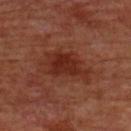workup = total-body-photography surveillance lesion; no biopsy | lesion size = ~6 mm (longest diameter) | image = total-body-photography crop, ~15 mm field of view | patient = male, aged 58 to 62 | anatomic site = the front of the torso | lighting = cross-polarized.